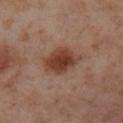<record>
  <biopsy_status>not biopsied; imaged during a skin examination</biopsy_status>
  <patient>
    <sex>female</sex>
    <age_approx>55</age_approx>
  </patient>
  <image>
    <source>total-body photography crop</source>
    <field_of_view_mm>15</field_of_view_mm>
  </image>
  <automated_metrics>
    <eccentricity>0.65</eccentricity>
    <shape_asymmetry>0.15</shape_asymmetry>
    <cielab_L>41</cielab_L>
    <cielab_a>22</cielab_a>
    <cielab_b>29</cielab_b>
    <vs_skin_darker_L>12.0</vs_skin_darker_L>
    <vs_skin_contrast_norm>10.0</vs_skin_contrast_norm>
    <border_irregularity_0_10>1.5</border_irregularity_0_10>
    <color_variation_0_10>3.5</color_variation_0_10>
    <peripheral_color_asymmetry>1.0</peripheral_color_asymmetry>
    <nevus_likeness_0_100>95</nevus_likeness_0_100>
    <lesion_detection_confidence_0_100>100</lesion_detection_confidence_0_100>
  </automated_metrics>
  <lighting>cross-polarized</lighting>
  <site>left lower leg</site>
</record>